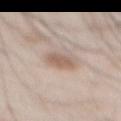<lesion>
  <biopsy_status>not biopsied; imaged during a skin examination</biopsy_status>
  <patient>
    <sex>male</sex>
    <age_approx>55</age_approx>
  </patient>
  <site>chest</site>
  <image>
    <source>total-body photography crop</source>
    <field_of_view_mm>15</field_of_view_mm>
  </image>
  <automated_metrics>
    <cielab_L>60</cielab_L>
    <cielab_a>15</cielab_a>
    <cielab_b>25</cielab_b>
    <vs_skin_darker_L>10.0</vs_skin_darker_L>
    <vs_skin_contrast_norm>6.5</vs_skin_contrast_norm>
    <nevus_likeness_0_100>95</nevus_likeness_0_100>
  </automated_metrics>
  <lighting>white-light</lighting>
  <lesion_size>
    <long_diameter_mm_approx>3.0</long_diameter_mm_approx>
  </lesion_size>
</lesion>